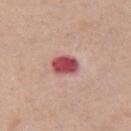notes: no biopsy performed (imaged during a skin exam)
site: the left upper arm
subject: male, approximately 45 years of age
diameter: ≈3.5 mm
lighting: white-light
imaging modality: total-body-photography crop, ~15 mm field of view